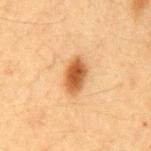Part of a total-body skin-imaging series; this lesion was reviewed on a skin check and was not flagged for biopsy.
Cropped from a total-body skin-imaging series; the visible field is about 15 mm.
A male subject in their mid- to late 60s.
The lesion is located on the mid back.
Automated image analysis of the tile measured a shape eccentricity near 0.85 and two-axis asymmetry of about 0.2. And it measured a border-irregularity rating of about 2/10 and radial color variation of about 1. The software also gave a lesion-detection confidence of about 100/100.
The tile uses cross-polarized illumination.
Longest diameter approximately 4 mm.